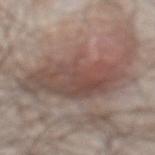<case>
<biopsy_status>not biopsied; imaged during a skin examination</biopsy_status>
</case>